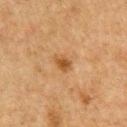biopsy status: no biopsy performed (imaged during a skin exam)
imaging modality: ~15 mm crop, total-body skin-cancer survey
body site: the chest
automated lesion analysis: an area of roughly 3.5 mm²; a mean CIELAB color near L≈42 a*≈19 b*≈34, roughly 9 lightness units darker than nearby skin, and a lesion-to-skin contrast of about 7.5 (normalized; higher = more distinct)
tile lighting: cross-polarized illumination
patient: male, aged 73–77
lesion size: ≈2.5 mm